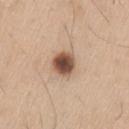Q: Was this lesion biopsied?
A: total-body-photography surveillance lesion; no biopsy